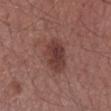This lesion was catalogued during total-body skin photography and was not selected for biopsy.
The tile uses white-light illumination.
The lesion-visualizer software estimated a lesion area of about 11 mm² and a symmetry-axis asymmetry near 0.15. And it measured an average lesion color of about L≈38 a*≈22 b*≈23 (CIELAB) and about 10 CIELAB-L* units darker than the surrounding skin. The software also gave a border-irregularity rating of about 2/10, a within-lesion color-variation index near 3/10, and a peripheral color-asymmetry measure near 1. The analysis additionally found a nevus-likeness score of about 65/100 and a detector confidence of about 100 out of 100 that the crop contains a lesion.
Cropped from a total-body skin-imaging series; the visible field is about 15 mm.
The lesion is on the right forearm.
A female patient, aged 53–57.